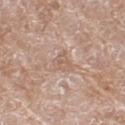image — total-body-photography crop, ~15 mm field of view
site — the right thigh
patient — female, approximately 75 years of age
TBP lesion metrics — a shape eccentricity near 0.75 and a shape-asymmetry score of about 0.45 (0 = symmetric); a mean CIELAB color near L≈60 a*≈18 b*≈27 and roughly 6 lightness units darker than nearby skin; border irregularity of about 4.5 on a 0–10 scale, a color-variation rating of about 2/10, and peripheral color asymmetry of about 0.5; a nevus-likeness score of about 0/100 and a detector confidence of about 90 out of 100 that the crop contains a lesion
illumination — white-light illumination